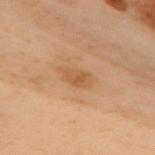biopsy_status: not biopsied; imaged during a skin examination
site: upper back
patient:
  sex: female
  age_approx: 60
lesion_size:
  long_diameter_mm_approx: 3.0
image:
  source: total-body photography crop
  field_of_view_mm: 15
automated_metrics:
  cielab_L: 48
  cielab_a: 19
  cielab_b: 34
  vs_skin_darker_L: 7.0
  vs_skin_contrast_norm: 6.0
  border_irregularity_0_10: 2.0
  peripheral_color_asymmetry: 1.0
  nevus_likeness_0_100: 5
  lesion_detection_confidence_0_100: 100
lighting: cross-polarized